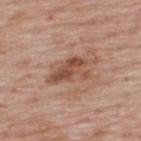Case summary:
* workup · no biopsy performed (imaged during a skin exam)
* lesion diameter · about 5 mm
* image source · total-body-photography crop, ~15 mm field of view
* body site · the upper back
* automated lesion analysis · a footprint of about 9.5 mm², an outline eccentricity of about 0.85 (0 = round, 1 = elongated), and two-axis asymmetry of about 0.4; a mean CIELAB color near L≈50 a*≈22 b*≈29 and a lesion–skin lightness drop of about 11; a border-irregularity index near 5/10, a within-lesion color-variation index near 4.5/10, and a peripheral color-asymmetry measure near 1.5; an automated nevus-likeness rating near 0 out of 100 and lesion-presence confidence of about 100/100
* subject · male, aged 63 to 67